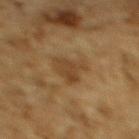This lesion was catalogued during total-body skin photography and was not selected for biopsy.
Automated tile analysis of the lesion measured a footprint of about 8.5 mm², a shape eccentricity near 0.8, and a shape-asymmetry score of about 0.4 (0 = symmetric). The analysis additionally found an average lesion color of about L≈36 a*≈14 b*≈31 (CIELAB) and a lesion-to-skin contrast of about 6.5 (normalized; higher = more distinct). The software also gave border irregularity of about 4.5 on a 0–10 scale, internal color variation of about 2 on a 0–10 scale, and peripheral color asymmetry of about 0.5. The analysis additionally found an automated nevus-likeness rating near 0 out of 100 and a lesion-detection confidence of about 90/100.
The recorded lesion diameter is about 4.5 mm.
On the upper back.
A roughly 15 mm field-of-view crop from a total-body skin photograph.
A male patient aged 83 or older.